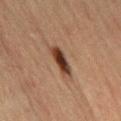  site: abdomen
  automated_metrics:
    cielab_L: 31
    cielab_a: 17
    cielab_b: 24
    vs_skin_darker_L: 13.0
    vs_skin_contrast_norm: 12.5
    border_irregularity_0_10: 3.0
    color_variation_0_10: 5.0
    peripheral_color_asymmetry: 1.0
  image:
    source: total-body photography crop
    field_of_view_mm: 15
  lesion_size:
    long_diameter_mm_approx: 4.5
  patient:
    sex: male
    age_approx: 65
  lighting: cross-polarized